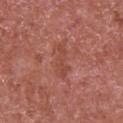Assessment:
Captured during whole-body skin photography for melanoma surveillance; the lesion was not biopsied.
Clinical summary:
A male subject aged approximately 65. Approximately 4.5 mm at its widest. The lesion is on the chest. A lesion tile, about 15 mm wide, cut from a 3D total-body photograph. This is a white-light tile.